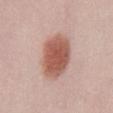The lesion was tiled from a total-body skin photograph and was not biopsied. This image is a 15 mm lesion crop taken from a total-body photograph. Automated image analysis of the tile measured a lesion area of about 19 mm², an outline eccentricity of about 0.7 (0 = round, 1 = elongated), and two-axis asymmetry of about 0.15. The analysis additionally found a lesion–skin lightness drop of about 14. The analysis additionally found border irregularity of about 1.5 on a 0–10 scale, a within-lesion color-variation index near 3.5/10, and a peripheral color-asymmetry measure near 1. The lesion's longest dimension is about 5.5 mm. A female patient, about 40 years old. This is a white-light tile. The lesion is located on the abdomen.